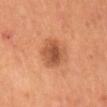workup = no biopsy performed (imaged during a skin exam)
imaging modality = total-body-photography crop, ~15 mm field of view
location = the mid back
lighting = cross-polarized illumination
subject = male, aged 53–57